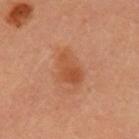notes = no biopsy performed (imaged during a skin exam) | image source = ~15 mm crop, total-body skin-cancer survey | patient = female, about 35 years old | body site = the arm | automated lesion analysis = a lesion area of about 8.5 mm², an eccentricity of roughly 0.8, and two-axis asymmetry of about 0.3; a mean CIELAB color near L≈50 a*≈26 b*≈36, about 8 CIELAB-L* units darker than the surrounding skin, and a normalized lesion–skin contrast near 6.5; a border-irregularity rating of about 3/10 and a color-variation rating of about 3.5/10; a nevus-likeness score of about 45/100.